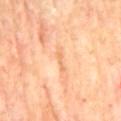Assessment: Recorded during total-body skin imaging; not selected for excision or biopsy. Context: Located on the mid back. This image is a 15 mm lesion crop taken from a total-body photograph. A male patient roughly 70 years of age.